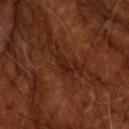Approximately 2.5 mm at its widest. A 15 mm close-up extracted from a 3D total-body photography capture. The patient is a male aged 63 to 67. Captured under cross-polarized illumination.